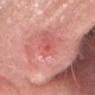Q: What did the biopsy show?
A: a nodular basal cell carcinoma — a malignant lesion